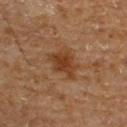This lesion was catalogued during total-body skin photography and was not selected for biopsy.
Captured under cross-polarized illumination.
About 4 mm across.
Cropped from a whole-body photographic skin survey; the tile spans about 15 mm.
From the upper back.
The patient is a male in their mid-60s.